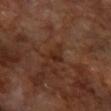No biopsy was performed on this lesion — it was imaged during a full skin examination and was not determined to be concerning.
The lesion is located on the right forearm.
A female patient aged 63 to 67.
Automated image analysis of the tile measured a mean CIELAB color near L≈27 a*≈20 b*≈26, a lesion–skin lightness drop of about 6, and a normalized border contrast of about 7. And it measured a nevus-likeness score of about 0/100 and lesion-presence confidence of about 100/100.
A 15 mm close-up tile from a total-body photography series done for melanoma screening.
This is a cross-polarized tile.